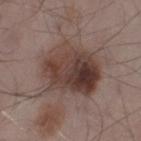Assessment: This lesion was catalogued during total-body skin photography and was not selected for biopsy. Clinical summary: Located on the left thigh. A male patient in their mid- to late 50s. Cropped from a whole-body photographic skin survey; the tile spans about 15 mm. Automated tile analysis of the lesion measured a lesion area of about 32 mm² and a shape eccentricity near 0.6. It also reported a lesion color around L≈40 a*≈16 b*≈21 in CIELAB and a normalized lesion–skin contrast near 9.5. And it measured a nevus-likeness score of about 5/100 and a detector confidence of about 100 out of 100 that the crop contains a lesion. The tile uses white-light illumination. Approximately 7 mm at its widest.